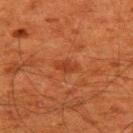site: back
lesion_size:
  long_diameter_mm_approx: 2.5
patient:
  sex: male
  age_approx: 60
image:
  source: total-body photography crop
  field_of_view_mm: 15
lighting: cross-polarized